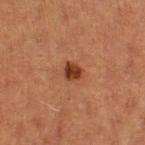Notes:
• biopsy status — imaged on a skin check; not biopsied
• anatomic site — the leg
• subject — female, roughly 40 years of age
• imaging modality — ~15 mm tile from a whole-body skin photo